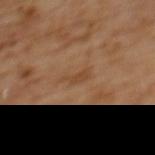  patient:
    sex: female
    age_approx: 55
  site: upper back
  lighting: cross-polarized
  lesion_size:
    long_diameter_mm_approx: 3.0
  image:
    source: total-body photography crop
    field_of_view_mm: 15